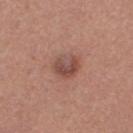Assessment:
The lesion was tiled from a total-body skin photograph and was not biopsied.
Image and clinical context:
The recorded lesion diameter is about 3.5 mm. The lesion is located on the right thigh. The tile uses white-light illumination. The subject is a female in their 30s. Cropped from a whole-body photographic skin survey; the tile spans about 15 mm.